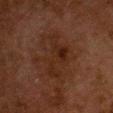This lesion was catalogued during total-body skin photography and was not selected for biopsy.
Automated image analysis of the tile measured a lesion area of about 12 mm², an eccentricity of roughly 0.85, and two-axis asymmetry of about 0.5. It also reported a color-variation rating of about 4/10 and peripheral color asymmetry of about 1. The analysis additionally found an automated nevus-likeness rating near 0 out of 100 and lesion-presence confidence of about 100/100.
This is a cross-polarized tile.
The subject is a female aged 48–52.
Cropped from a whole-body photographic skin survey; the tile spans about 15 mm.
Approximately 5.5 mm at its widest.
On the chest.